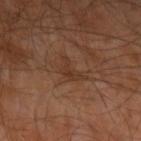Assessment:
This lesion was catalogued during total-body skin photography and was not selected for biopsy.
Background:
The lesion-visualizer software estimated an outline eccentricity of about 0.75 (0 = round, 1 = elongated) and two-axis asymmetry of about 0.55. A male patient aged 63–67. The tile uses cross-polarized illumination. Located on the left upper arm. A 15 mm crop from a total-body photograph taken for skin-cancer surveillance.